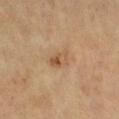Q: Is there a histopathology result?
A: catalogued during a skin exam; not biopsied
Q: Lesion location?
A: the abdomen
Q: How large is the lesion?
A: ~3 mm (longest diameter)
Q: What are the patient's age and sex?
A: male, aged 63 to 67
Q: What did automated image analysis measure?
A: a footprint of about 4.5 mm², a shape eccentricity near 0.8, and two-axis asymmetry of about 0.3; a peripheral color-asymmetry measure near 2.5
Q: How was the tile lit?
A: cross-polarized
Q: What kind of image is this?
A: 15 mm crop, total-body photography